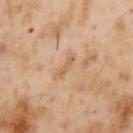Captured during whole-body skin photography for melanoma surveillance; the lesion was not biopsied.
A male subject, in their mid-50s.
A close-up tile cropped from a whole-body skin photograph, about 15 mm across.
From the arm.
Captured under cross-polarized illumination.